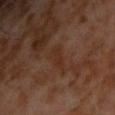Clinical impression: Part of a total-body skin-imaging series; this lesion was reviewed on a skin check and was not flagged for biopsy. Clinical summary: From the chest. A 15 mm close-up extracted from a 3D total-body photography capture. The patient is a male aged 58–62.